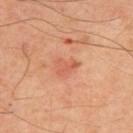Part of a total-body skin-imaging series; this lesion was reviewed on a skin check and was not flagged for biopsy.
A 15 mm crop from a total-body photograph taken for skin-cancer surveillance.
Automated tile analysis of the lesion measured a lesion area of about 4 mm² and an outline eccentricity of about 0.75 (0 = round, 1 = elongated). The software also gave a within-lesion color-variation index near 2/10 and peripheral color asymmetry of about 1.
Measured at roughly 2.5 mm in maximum diameter.
The lesion is located on the upper back.
The subject is a male approximately 50 years of age.